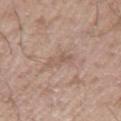The lesion was tiled from a total-body skin photograph and was not biopsied.
On the arm.
The lesion-visualizer software estimated a footprint of about 3.5 mm² and a shape-asymmetry score of about 0.4 (0 = symmetric). And it measured about 7 CIELAB-L* units darker than the surrounding skin and a lesion-to-skin contrast of about 5 (normalized; higher = more distinct). The software also gave a within-lesion color-variation index near 0/10 and peripheral color asymmetry of about 0.
The subject is a male aged 68 to 72.
Cropped from a total-body skin-imaging series; the visible field is about 15 mm.
Longest diameter approximately 3 mm.
Imaged with white-light lighting.